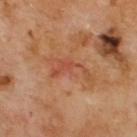Q: Was a biopsy performed?
A: imaged on a skin check; not biopsied
Q: What lighting was used for the tile?
A: cross-polarized illumination
Q: What is the anatomic site?
A: the upper back
Q: What did automated image analysis measure?
A: an area of roughly 6.5 mm², a shape eccentricity near 0.9, and a symmetry-axis asymmetry near 0.7; a border-irregularity index near 9.5/10, a color-variation rating of about 1.5/10, and radial color variation of about 0.5
Q: How large is the lesion?
A: ≈5 mm
Q: What are the patient's age and sex?
A: male, aged 68 to 72
Q: What is the imaging modality?
A: ~15 mm tile from a whole-body skin photo